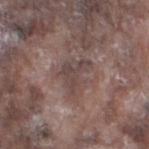Clinical impression: This lesion was catalogued during total-body skin photography and was not selected for biopsy. Clinical summary: A 15 mm crop from a total-body photograph taken for skin-cancer surveillance. Imaged with white-light lighting. A male patient approximately 75 years of age. The lesion is on the left thigh. The total-body-photography lesion software estimated a mean CIELAB color near L≈43 a*≈15 b*≈18. The software also gave a border-irregularity index near 6/10. The software also gave a detector confidence of about 70 out of 100 that the crop contains a lesion. About 4 mm across.